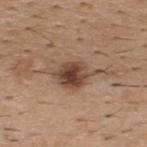<tbp_lesion>
  <biopsy_status>not biopsied; imaged during a skin examination</biopsy_status>
  <patient>
    <sex>male</sex>
    <age_approx>40</age_approx>
  </patient>
  <automated_metrics>
    <eccentricity>0.85</eccentricity>
    <shape_asymmetry>0.35</shape_asymmetry>
    <border_irregularity_0_10>5.0</border_irregularity_0_10>
    <color_variation_0_10>8.0</color_variation_0_10>
    <nevus_likeness_0_100>95</nevus_likeness_0_100>
    <lesion_detection_confidence_0_100>100</lesion_detection_confidence_0_100>
  </automated_metrics>
  <lighting>white-light</lighting>
  <lesion_size>
    <long_diameter_mm_approx>7.5</long_diameter_mm_approx>
  </lesion_size>
  <image>
    <source>total-body photography crop</source>
    <field_of_view_mm>15</field_of_view_mm>
  </image>
  <site>back</site>
</tbp_lesion>